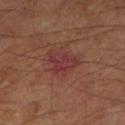Clinical impression: This lesion was catalogued during total-body skin photography and was not selected for biopsy. Context: The lesion's longest dimension is about 4 mm. A roughly 15 mm field-of-view crop from a total-body skin photograph. Imaged with cross-polarized lighting. A male patient, aged approximately 65.